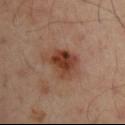Q: Was this lesion biopsied?
A: imaged on a skin check; not biopsied
Q: Lesion location?
A: the left arm
Q: What is the lesion's diameter?
A: ≈4 mm
Q: What did automated image analysis measure?
A: an area of roughly 10 mm², an eccentricity of roughly 0.5, and a shape-asymmetry score of about 0.35 (0 = symmetric); border irregularity of about 4 on a 0–10 scale and a color-variation rating of about 5.5/10; a classifier nevus-likeness of about 85/100 and lesion-presence confidence of about 100/100
Q: What kind of image is this?
A: ~15 mm crop, total-body skin-cancer survey
Q: Who is the patient?
A: male, aged approximately 50
Q: Illumination type?
A: cross-polarized illumination